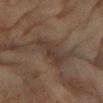workup — no biopsy performed (imaged during a skin exam)
subject — female, aged approximately 80
tile lighting — cross-polarized illumination
body site — the right forearm
image — ~15 mm crop, total-body skin-cancer survey
size — about 4 mm